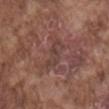<record>
<biopsy_status>not biopsied; imaged during a skin examination</biopsy_status>
<patient>
  <sex>male</sex>
  <age_approx>75</age_approx>
</patient>
<image>
  <source>total-body photography crop</source>
  <field_of_view_mm>15</field_of_view_mm>
</image>
<lesion_size>
  <long_diameter_mm_approx>4.0</long_diameter_mm_approx>
</lesion_size>
<automated_metrics>
  <cielab_L>40</cielab_L>
  <cielab_a>19</cielab_a>
  <cielab_b>21</cielab_b>
  <vs_skin_darker_L>6.0</vs_skin_darker_L>
  <vs_skin_contrast_norm>5.0</vs_skin_contrast_norm>
  <color_variation_0_10>0.0</color_variation_0_10>
  <peripheral_color_asymmetry>0.0</peripheral_color_asymmetry>
</automated_metrics>
<site>mid back</site>
<lighting>white-light</lighting>
</record>